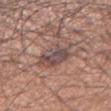Captured during whole-body skin photography for melanoma surveillance; the lesion was not biopsied.